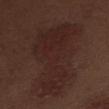follow-up — catalogued during a skin exam; not biopsied
subject — male, about 70 years old
imaging modality — 15 mm crop, total-body photography
anatomic site — the mid back
diameter — about 11.5 mm